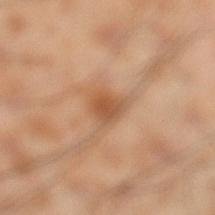| key | value |
|---|---|
| follow-up | total-body-photography surveillance lesion; no biopsy |
| body site | the left lower leg |
| image source | total-body-photography crop, ~15 mm field of view |
| subject | male, aged approximately 55 |